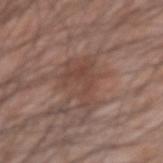Q: Was this lesion biopsied?
A: total-body-photography surveillance lesion; no biopsy
Q: Lesion location?
A: the arm
Q: Who is the patient?
A: male, aged around 45
Q: What kind of image is this?
A: ~15 mm tile from a whole-body skin photo
Q: How large is the lesion?
A: about 5.5 mm